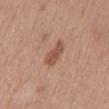Findings:
• notes · catalogued during a skin exam; not biopsied
• image-analysis metrics · a lesion area of about 5.5 mm², a shape eccentricity near 0.85, and a symmetry-axis asymmetry near 0.3; a nevus-likeness score of about 55/100
• lesion size · ~3.5 mm (longest diameter)
• tile lighting · white-light
• subject · female, aged 53–57
• image source · total-body-photography crop, ~15 mm field of view
• location · the back